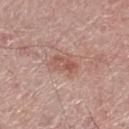| feature | finding |
|---|---|
| follow-up | catalogued during a skin exam; not biopsied |
| image source | 15 mm crop, total-body photography |
| illumination | white-light |
| patient | male, approximately 70 years of age |
| automated metrics | a classifier nevus-likeness of about 10/100 |
| location | the right lower leg |
| size | ~3 mm (longest diameter) |